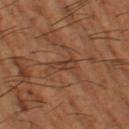Case summary:
- biopsy status: total-body-photography surveillance lesion; no biopsy
- lighting: cross-polarized illumination
- lesion size: ~3.5 mm (longest diameter)
- image source: total-body-photography crop, ~15 mm field of view
- subject: male, in their mid- to late 60s
- site: the left thigh